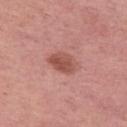A close-up tile cropped from a whole-body skin photograph, about 15 mm across. The patient is a female aged around 55. Captured under white-light illumination. The recorded lesion diameter is about 3.5 mm. Located on the left thigh.